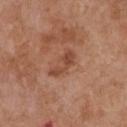Q: Was this lesion biopsied?
A: total-body-photography surveillance lesion; no biopsy
Q: What is the imaging modality?
A: ~15 mm crop, total-body skin-cancer survey
Q: Illumination type?
A: white-light illumination
Q: What is the anatomic site?
A: the front of the torso
Q: Lesion size?
A: ≈4 mm
Q: What are the patient's age and sex?
A: female, about 75 years old